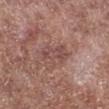Imaged during a routine full-body skin examination; the lesion was not biopsied and no histopathology is available.
This image is a 15 mm lesion crop taken from a total-body photograph.
Longest diameter approximately 4 mm.
The lesion is located on the right lower leg.
Automated image analysis of the tile measured an area of roughly 8 mm², an eccentricity of roughly 0.8, and two-axis asymmetry of about 0.3. The software also gave a lesion color around L≈48 a*≈21 b*≈22 in CIELAB, roughly 8 lightness units darker than nearby skin, and a normalized border contrast of about 6. And it measured border irregularity of about 4 on a 0–10 scale, a within-lesion color-variation index near 3/10, and a peripheral color-asymmetry measure near 1. And it measured an automated nevus-likeness rating near 0 out of 100 and lesion-presence confidence of about 100/100.
A male subject, approximately 55 years of age.